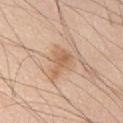Impression:
Recorded during total-body skin imaging; not selected for excision or biopsy.
Acquisition and patient details:
A male subject, aged around 60. A 15 mm close-up extracted from a 3D total-body photography capture. The recorded lesion diameter is about 4 mm. The lesion is located on the front of the torso. Imaged with white-light lighting.